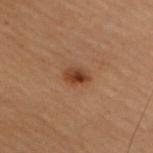{"image": {"source": "total-body photography crop", "field_of_view_mm": 15}, "patient": {"sex": "female", "age_approx": 50}, "site": "upper back", "lesion_size": {"long_diameter_mm_approx": 2.5}}